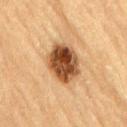biopsy status: total-body-photography surveillance lesion; no biopsy | imaging modality: total-body-photography crop, ~15 mm field of view | body site: the lower back | subject: male, in their mid-80s or older.